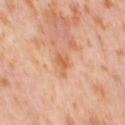Q: How large is the lesion?
A: about 3 mm
Q: What is the anatomic site?
A: the leg
Q: Automated lesion metrics?
A: an outline eccentricity of about 0.85 (0 = round, 1 = elongated) and a shape-asymmetry score of about 0.4 (0 = symmetric)
Q: How was this image acquired?
A: 15 mm crop, total-body photography
Q: Who is the patient?
A: female, aged around 55
Q: Illumination type?
A: cross-polarized